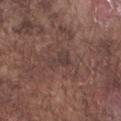biopsy_status: not biopsied; imaged during a skin examination
site: right forearm
image:
  source: total-body photography crop
  field_of_view_mm: 15
patient:
  sex: male
  age_approx: 75